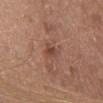Notes:
– notes · total-body-photography surveillance lesion; no biopsy
– image · 15 mm crop, total-body photography
– lesion size · about 3 mm
– lighting · white-light illumination
– subject · female, roughly 50 years of age
– body site · the chest